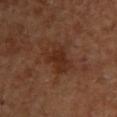The lesion was photographed on a routine skin check and not biopsied; there is no pathology result. Captured under cross-polarized illumination. The subject is a female aged 58–62. An algorithmic analysis of the crop reported a footprint of about 9.5 mm², an eccentricity of roughly 0.75, and a symmetry-axis asymmetry near 0.3. It also reported a mean CIELAB color near L≈30 a*≈21 b*≈29, roughly 7 lightness units darker than nearby skin, and a normalized lesion–skin contrast near 7. Measured at roughly 4.5 mm in maximum diameter. A region of skin cropped from a whole-body photographic capture, roughly 15 mm wide. Located on the arm.